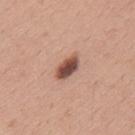Clinical impression:
Captured during whole-body skin photography for melanoma surveillance; the lesion was not biopsied.
Clinical summary:
A close-up tile cropped from a whole-body skin photograph, about 15 mm across. A male patient, approximately 30 years of age. The lesion is located on the upper back.